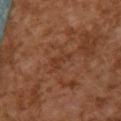Imaged during a routine full-body skin examination; the lesion was not biopsied and no histopathology is available.
A female patient, roughly 60 years of age.
The total-body-photography lesion software estimated an outline eccentricity of about 0.9 (0 = round, 1 = elongated). The software also gave a lesion color around L≈31 a*≈20 b*≈27 in CIELAB, about 5 CIELAB-L* units darker than the surrounding skin, and a lesion-to-skin contrast of about 5 (normalized; higher = more distinct). The analysis additionally found a border-irregularity index near 5/10 and a within-lesion color-variation index near 0/10.
Cropped from a total-body skin-imaging series; the visible field is about 15 mm.
Located on the upper back.